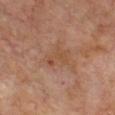Clinical impression:
No biopsy was performed on this lesion — it was imaged during a full skin examination and was not determined to be concerning.
Background:
From the chest. An algorithmic analysis of the crop reported an average lesion color of about L≈47 a*≈21 b*≈31 (CIELAB) and a lesion-to-skin contrast of about 5.5 (normalized; higher = more distinct). And it measured a color-variation rating of about 3/10. And it measured a nevus-likeness score of about 0/100 and a detector confidence of about 100 out of 100 that the crop contains a lesion. The tile uses cross-polarized illumination. A male subject approximately 70 years of age. Cropped from a whole-body photographic skin survey; the tile spans about 15 mm.